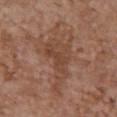Impression: Part of a total-body skin-imaging series; this lesion was reviewed on a skin check and was not flagged for biopsy. Acquisition and patient details: A 15 mm close-up extracted from a 3D total-body photography capture. About 7 mm across. Imaged with white-light lighting. The lesion is located on the chest. The subject is a female aged approximately 65.